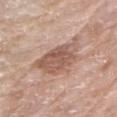This lesion was catalogued during total-body skin photography and was not selected for biopsy. From the arm. The lesion-visualizer software estimated an area of roughly 16 mm². The software also gave about 11 CIELAB-L* units darker than the surrounding skin and a lesion-to-skin contrast of about 7.5 (normalized; higher = more distinct). And it measured border irregularity of about 3.5 on a 0–10 scale, internal color variation of about 4 on a 0–10 scale, and radial color variation of about 1.5. It also reported a nevus-likeness score of about 0/100 and a detector confidence of about 100 out of 100 that the crop contains a lesion. A roughly 15 mm field-of-view crop from a total-body skin photograph. The lesion's longest dimension is about 6 mm. A male patient, aged 78–82.